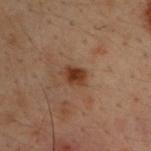Assessment: Imaged during a routine full-body skin examination; the lesion was not biopsied and no histopathology is available. Context: A region of skin cropped from a whole-body photographic capture, roughly 15 mm wide. The recorded lesion diameter is about 2.5 mm. Captured under cross-polarized illumination. On the upper back. Automated image analysis of the tile measured a footprint of about 4 mm², an eccentricity of roughly 0.7, and a shape-asymmetry score of about 0.2 (0 = symmetric). And it measured a nevus-likeness score of about 95/100. A male subject approximately 30 years of age.